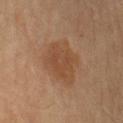notes: imaged on a skin check; not biopsied
lighting: cross-polarized illumination
lesion size: ≈5.5 mm
acquisition: ~15 mm crop, total-body skin-cancer survey
body site: the left upper arm
subject: male, about 55 years old